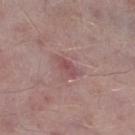follow-up: imaged on a skin check; not biopsied
body site: the right lower leg
subject: male, aged 73 to 77
acquisition: 15 mm crop, total-body photography
tile lighting: white-light